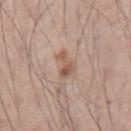{"biopsy_status": "not biopsied; imaged during a skin examination", "lighting": "white-light", "patient": {"sex": "male", "age_approx": 70}, "lesion_size": {"long_diameter_mm_approx": 3.0}, "image": {"source": "total-body photography crop", "field_of_view_mm": 15}, "site": "left upper arm"}